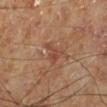Part of a total-body skin-imaging series; this lesion was reviewed on a skin check and was not flagged for biopsy. A lesion tile, about 15 mm wide, cut from a 3D total-body photograph. About 3 mm across. Captured under cross-polarized illumination. The patient is a male aged around 70. From the left lower leg.